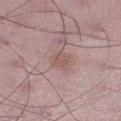Q: Was a biopsy performed?
A: total-body-photography surveillance lesion; no biopsy
Q: Lesion location?
A: the right lower leg
Q: What kind of image is this?
A: ~15 mm tile from a whole-body skin photo
Q: Illumination type?
A: white-light illumination
Q: What did automated image analysis measure?
A: an area of roughly 4.5 mm², an outline eccentricity of about 0.5 (0 = round, 1 = elongated), and two-axis asymmetry of about 0.25; a border-irregularity index near 2.5/10
Q: Lesion size?
A: ~2.5 mm (longest diameter)
Q: Who is the patient?
A: male, aged 48 to 52